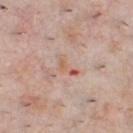Cropped from a whole-body photographic skin survey; the tile spans about 15 mm.
Longest diameter approximately 3.5 mm.
A male patient aged approximately 40.
From the chest.